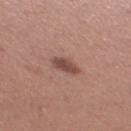• follow-up · total-body-photography surveillance lesion; no biopsy
• patient · female, aged 28–32
• diameter · ~3 mm (longest diameter)
• site · the left thigh
• illumination · white-light
• image source · 15 mm crop, total-body photography